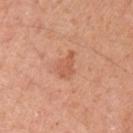Clinical summary:
Approximately 3.5 mm at its widest. Cropped from a whole-body photographic skin survey; the tile spans about 15 mm. The lesion-visualizer software estimated roughly 8 lightness units darker than nearby skin and a normalized lesion–skin contrast near 5.5. The software also gave an automated nevus-likeness rating near 15 out of 100 and a detector confidence of about 100 out of 100 that the crop contains a lesion. Imaged with white-light lighting. A female patient roughly 40 years of age. The lesion is located on the left upper arm.